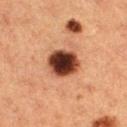biopsy status — imaged on a skin check; not biopsied | automated metrics — internal color variation of about 7 on a 0–10 scale and peripheral color asymmetry of about 1.5; a nevus-likeness score of about 75/100 and a lesion-detection confidence of about 100/100 | image source — ~15 mm crop, total-body skin-cancer survey | site — the left thigh | patient — female, approximately 40 years of age | lighting — cross-polarized.